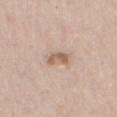biopsy_status: not biopsied; imaged during a skin examination